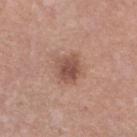<tbp_lesion>
  <site>left lower leg</site>
  <image>
    <source>total-body photography crop</source>
    <field_of_view_mm>15</field_of_view_mm>
  </image>
  <patient>
    <sex>female</sex>
    <age_approx>30</age_approx>
  </patient>
  <lesion_size>
    <long_diameter_mm_approx>3.0</long_diameter_mm_approx>
  </lesion_size>
  <lighting>white-light</lighting>
</tbp_lesion>